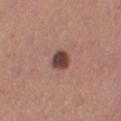Q: Was this lesion biopsied?
A: no biopsy performed (imaged during a skin exam)
Q: What is the anatomic site?
A: the right thigh
Q: Patient demographics?
A: female, approximately 25 years of age
Q: How was this image acquired?
A: ~15 mm tile from a whole-body skin photo